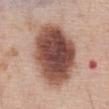Captured under white-light illumination.
The subject is a male aged approximately 70.
From the abdomen.
A lesion tile, about 15 mm wide, cut from a 3D total-body photograph.